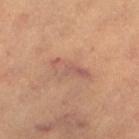| field | value |
|---|---|
| notes | no biopsy performed (imaged during a skin exam) |